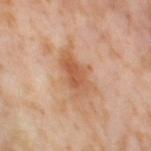No biopsy was performed on this lesion — it was imaged during a full skin examination and was not determined to be concerning. The lesion is on the right thigh. A female subject, aged 53–57. This image is a 15 mm lesion crop taken from a total-body photograph.